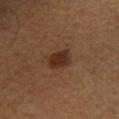Q: Is there a histopathology result?
A: imaged on a skin check; not biopsied
Q: Lesion size?
A: ~3.5 mm (longest diameter)
Q: Who is the patient?
A: male, about 55 years old
Q: Where on the body is the lesion?
A: the right lower leg
Q: Automated lesion metrics?
A: an area of roughly 7 mm², an outline eccentricity of about 0.6 (0 = round, 1 = elongated), and a shape-asymmetry score of about 0.2 (0 = symmetric); an average lesion color of about L≈26 a*≈17 b*≈25 (CIELAB), about 8 CIELAB-L* units darker than the surrounding skin, and a normalized lesion–skin contrast near 8.5; a border-irregularity rating of about 2/10 and a within-lesion color-variation index near 3/10
Q: What is the imaging modality?
A: total-body-photography crop, ~15 mm field of view
Q: How was the tile lit?
A: cross-polarized